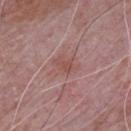notes = no biopsy performed (imaged during a skin exam) | acquisition = ~15 mm crop, total-body skin-cancer survey | site = the chest | subject = male, aged around 65.